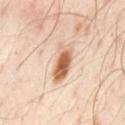biopsy_status: not biopsied; imaged during a skin examination
lighting: cross-polarized
automated_metrics:
  vs_skin_darker_L: 16.0
  border_irregularity_0_10: 3.5
  color_variation_0_10: 8.0
  peripheral_color_asymmetry: 2.0
site: front of the torso
lesion_size:
  long_diameter_mm_approx: 5.0
patient:
  sex: male
  age_approx: 50
image:
  source: total-body photography crop
  field_of_view_mm: 15